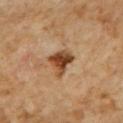workup = no biopsy performed (imaged during a skin exam)
patient = male, in their 60s
lesion size = ≈3.5 mm
tile lighting = cross-polarized illumination
site = the chest
imaging modality = total-body-photography crop, ~15 mm field of view